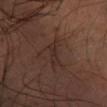follow-up = catalogued during a skin exam; not biopsied | acquisition = 15 mm crop, total-body photography | diameter = about 2.5 mm | subject = male, in their mid-40s | location = the left forearm | lighting = cross-polarized illumination.